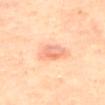Q: Was a biopsy performed?
A: imaged on a skin check; not biopsied
Q: Who is the patient?
A: female, about 60 years old
Q: What kind of image is this?
A: ~15 mm crop, total-body skin-cancer survey
Q: Lesion location?
A: the upper back